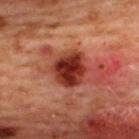{
  "biopsy_status": "not biopsied; imaged during a skin examination",
  "site": "upper back",
  "lighting": "cross-polarized",
  "image": {
    "source": "total-body photography crop",
    "field_of_view_mm": 15
  },
  "lesion_size": {
    "long_diameter_mm_approx": 4.0
  },
  "patient": {
    "sex": "female",
    "age_approx": 45
  }
}